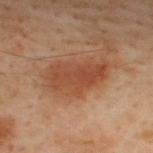Notes:
– notes · no biopsy performed (imaged during a skin exam)
– lesion size · ~6.5 mm (longest diameter)
– site · the upper back
– image · 15 mm crop, total-body photography
– tile lighting · cross-polarized illumination
– subject · male, in their 50s